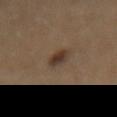The total-body-photography lesion software estimated a footprint of about 4 mm² and an eccentricity of roughly 0.75. The analysis additionally found a mean CIELAB color near L≈32 a*≈13 b*≈22, a lesion–skin lightness drop of about 9, and a normalized lesion–skin contrast near 9.5. The analysis additionally found a peripheral color-asymmetry measure near 2. The software also gave a nevus-likeness score of about 100/100. About 2.5 mm across. The patient is a female in their 60s. The lesion is on the back. Captured under cross-polarized illumination. A lesion tile, about 15 mm wide, cut from a 3D total-body photograph.